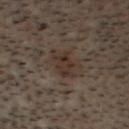Imaged during a routine full-body skin examination; the lesion was not biopsied and no histopathology is available. A male subject about 30 years old. Imaged with cross-polarized lighting. On the head or neck. A close-up tile cropped from a whole-body skin photograph, about 15 mm across.